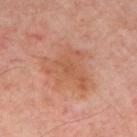Clinical impression: This lesion was catalogued during total-body skin photography and was not selected for biopsy. Clinical summary: A subject aged 53 to 57. Captured under cross-polarized illumination. A region of skin cropped from a whole-body photographic capture, roughly 15 mm wide. An algorithmic analysis of the crop reported an eccentricity of roughly 0.55 and a shape-asymmetry score of about 0.5 (0 = symmetric). And it measured roughly 7 lightness units darker than nearby skin. The software also gave a border-irregularity rating of about 6/10, a within-lesion color-variation index near 3/10, and a peripheral color-asymmetry measure near 1. Located on the mid back.